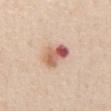Part of a total-body skin-imaging series; this lesion was reviewed on a skin check and was not flagged for biopsy. Cropped from a total-body skin-imaging series; the visible field is about 15 mm. A male patient aged approximately 60. Longest diameter approximately 4 mm. The lesion is located on the abdomen. This is a white-light tile.